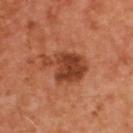| field | value |
|---|---|
| image-analysis metrics | a lesion area of about 14 mm² and an outline eccentricity of about 0.8 (0 = round, 1 = elongated); a mean CIELAB color near L≈42 a*≈28 b*≈34, about 12 CIELAB-L* units darker than the surrounding skin, and a lesion-to-skin contrast of about 9 (normalized; higher = more distinct); a border-irregularity index near 5.5/10, a within-lesion color-variation index near 4/10, and a peripheral color-asymmetry measure near 1.5; a classifier nevus-likeness of about 35/100 and a lesion-detection confidence of about 100/100 |
| acquisition | total-body-photography crop, ~15 mm field of view |
| body site | the upper back |
| tile lighting | cross-polarized |
| patient | male, aged around 50 |